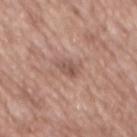Background:
From the back. The total-body-photography lesion software estimated an area of roughly 3.5 mm², an outline eccentricity of about 0.7 (0 = round, 1 = elongated), and two-axis asymmetry of about 0.3. And it measured border irregularity of about 3 on a 0–10 scale, a within-lesion color-variation index near 2.5/10, and a peripheral color-asymmetry measure near 1. A female patient about 75 years old. A region of skin cropped from a whole-body photographic capture, roughly 15 mm wide. The recorded lesion diameter is about 2.5 mm. Captured under white-light illumination.